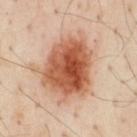Notes:
– workup — catalogued during a skin exam; not biopsied
– location — the chest
– subject — male, about 55 years old
– acquisition — total-body-photography crop, ~15 mm field of view